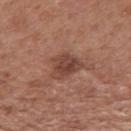Assessment: Part of a total-body skin-imaging series; this lesion was reviewed on a skin check and was not flagged for biopsy. Acquisition and patient details: This is a white-light tile. This image is a 15 mm lesion crop taken from a total-body photograph. Longest diameter approximately 4 mm. A male patient, approximately 70 years of age. From the mid back.